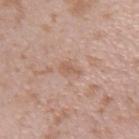This lesion was catalogued during total-body skin photography and was not selected for biopsy. Imaged with white-light lighting. A male patient in their mid-20s. Measured at roughly 2.5 mm in maximum diameter. A lesion tile, about 15 mm wide, cut from a 3D total-body photograph. The lesion is on the arm.